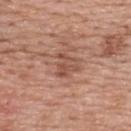Q: Is there a histopathology result?
A: no biopsy performed (imaged during a skin exam)
Q: What kind of image is this?
A: total-body-photography crop, ~15 mm field of view
Q: Where on the body is the lesion?
A: the upper back
Q: What are the patient's age and sex?
A: female, aged around 60